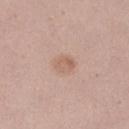The lesion was photographed on a routine skin check and not biopsied; there is no pathology result.